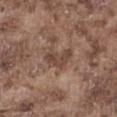The lesion was tiled from a total-body skin photograph and was not biopsied. An algorithmic analysis of the crop reported an area of roughly 6 mm², an outline eccentricity of about 0.75 (0 = round, 1 = elongated), and a symmetry-axis asymmetry near 0.55. And it measured a mean CIELAB color near L≈44 a*≈18 b*≈25 and a lesion-to-skin contrast of about 7.5 (normalized; higher = more distinct). A region of skin cropped from a whole-body photographic capture, roughly 15 mm wide. The lesion is on the abdomen. A male subject, aged 73 to 77.